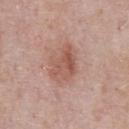Clinical impression:
This lesion was catalogued during total-body skin photography and was not selected for biopsy.
Acquisition and patient details:
About 4.5 mm across. A close-up tile cropped from a whole-body skin photograph, about 15 mm across. The patient is a male aged 53 to 57. From the chest.